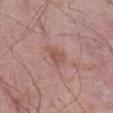biopsy status: imaged on a skin check; not biopsied | image-analysis metrics: a footprint of about 4 mm² and a shape eccentricity near 0.75; a classifier nevus-likeness of about 0/100 and a detector confidence of about 100 out of 100 that the crop contains a lesion | anatomic site: the abdomen | acquisition: 15 mm crop, total-body photography | subject: male, in their 70s | illumination: white-light illumination | lesion size: ≈3 mm.